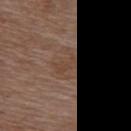Clinical impression: The lesion was tiled from a total-body skin photograph and was not biopsied. Clinical summary: This is a white-light tile. About 2.5 mm across. The lesion-visualizer software estimated a lesion area of about 3.5 mm², an outline eccentricity of about 0.9 (0 = round, 1 = elongated), and a symmetry-axis asymmetry near 0.35. It also reported a border-irregularity rating of about 4.5/10, a within-lesion color-variation index near 1/10, and radial color variation of about 0.5. A region of skin cropped from a whole-body photographic capture, roughly 15 mm wide. A female patient, roughly 70 years of age. Located on the upper back.